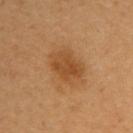| key | value |
|---|---|
| workup | total-body-photography surveillance lesion; no biopsy |
| site | the right upper arm |
| lighting | cross-polarized illumination |
| diameter | ~4.5 mm (longest diameter) |
| automated lesion analysis | an average lesion color of about L≈47 a*≈22 b*≈39 (CIELAB), about 9 CIELAB-L* units darker than the surrounding skin, and a normalized lesion–skin contrast near 7; a border-irregularity rating of about 2/10 and internal color variation of about 3.5 on a 0–10 scale; an automated nevus-likeness rating near 75 out of 100 and a detector confidence of about 100 out of 100 that the crop contains a lesion |
| patient | female, in their mid-30s |
| image | ~15 mm tile from a whole-body skin photo |